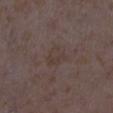Q: Was this lesion biopsied?
A: total-body-photography surveillance lesion; no biopsy
Q: What kind of image is this?
A: total-body-photography crop, ~15 mm field of view
Q: Automated lesion metrics?
A: an area of roughly 5 mm² and a shape-asymmetry score of about 0.3 (0 = symmetric); a mean CIELAB color near L≈37 a*≈12 b*≈19 and roughly 4 lightness units darker than nearby skin
Q: Who is the patient?
A: female, aged around 35
Q: What is the lesion's diameter?
A: ≈2.5 mm
Q: Where on the body is the lesion?
A: the leg
Q: What lighting was used for the tile?
A: white-light